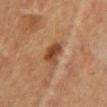Part of a total-body skin-imaging series; this lesion was reviewed on a skin check and was not flagged for biopsy.
A lesion tile, about 15 mm wide, cut from a 3D total-body photograph.
A male patient, aged approximately 60.
On the chest.